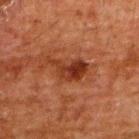follow-up = total-body-photography surveillance lesion; no biopsy
subject = male, approximately 60 years of age
site = the upper back
image = 15 mm crop, total-body photography
lesion size = ≈4.5 mm